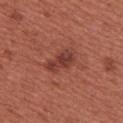Clinical impression:
Imaged during a routine full-body skin examination; the lesion was not biopsied and no histopathology is available.
Acquisition and patient details:
A female subject aged approximately 25. A 15 mm crop from a total-body photograph taken for skin-cancer surveillance. The lesion is on the upper back.